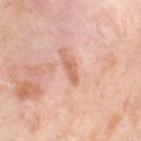| feature | finding |
|---|---|
| biopsy status | imaged on a skin check; not biopsied |
| size | ≈3 mm |
| body site | the right thigh |
| subject | female, in their 50s |
| image | total-body-photography crop, ~15 mm field of view |
| TBP lesion metrics | about 10 CIELAB-L* units darker than the surrounding skin and a normalized lesion–skin contrast near 7.5 |